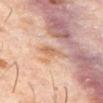Part of a total-body skin-imaging series; this lesion was reviewed on a skin check and was not flagged for biopsy. The lesion's longest dimension is about 3 mm. Located on the abdomen. A close-up tile cropped from a whole-body skin photograph, about 15 mm across. A male patient, approximately 60 years of age. This is a cross-polarized tile.